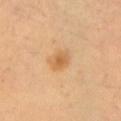biopsy status=imaged on a skin check; not biopsied | subject=male, aged 53–57 | automated metrics=a lesion area of about 5 mm², a shape eccentricity near 0.7, and two-axis asymmetry of about 0.2; a within-lesion color-variation index near 3.5/10 and peripheral color asymmetry of about 1 | body site=the left upper arm | lighting=cross-polarized | image=~15 mm crop, total-body skin-cancer survey.